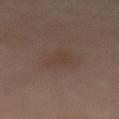biopsy status — imaged on a skin check; not biopsied | size — ≈3.5 mm | subject — female, in their 50s | location — the front of the torso | acquisition — 15 mm crop, total-body photography | illumination — cross-polarized.